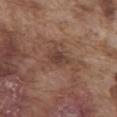The lesion was photographed on a routine skin check and not biopsied; there is no pathology result. A close-up tile cropped from a whole-body skin photograph, about 15 mm across. Located on the abdomen. A male patient in their mid- to late 70s. The recorded lesion diameter is about 3 mm.